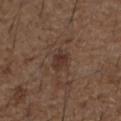No biopsy was performed on this lesion — it was imaged during a full skin examination and was not determined to be concerning. From the right forearm. This is a white-light tile. Cropped from a total-body skin-imaging series; the visible field is about 15 mm. The subject is a male about 50 years old. Automated image analysis of the tile measured a lesion area of about 4.5 mm² and an eccentricity of roughly 0.65. The software also gave a border-irregularity rating of about 2/10, a color-variation rating of about 2.5/10, and peripheral color asymmetry of about 1.